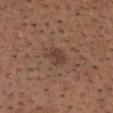workup — imaged on a skin check; not biopsied
site — the head or neck
subject — male, roughly 65 years of age
image-analysis metrics — a lesion area of about 5 mm², an outline eccentricity of about 0.75 (0 = round, 1 = elongated), and a symmetry-axis asymmetry near 0.25; an average lesion color of about L≈41 a*≈18 b*≈26 (CIELAB) and roughly 8 lightness units darker than nearby skin; a lesion-detection confidence of about 100/100
image — ~15 mm crop, total-body skin-cancer survey
tile lighting — white-light illumination